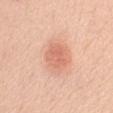Captured during whole-body skin photography for melanoma surveillance; the lesion was not biopsied. The lesion is located on the abdomen. The patient is a female in their 70s. A 15 mm close-up tile from a total-body photography series done for melanoma screening. The tile uses white-light illumination.